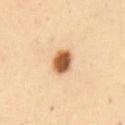The lesion was photographed on a routine skin check and not biopsied; there is no pathology result.
The lesion's longest dimension is about 2.5 mm.
On the abdomen.
This is a cross-polarized tile.
A female subject, about 50 years old.
A 15 mm close-up extracted from a 3D total-body photography capture.
An algorithmic analysis of the crop reported a lesion color around L≈50 a*≈19 b*≈35 in CIELAB, a lesion–skin lightness drop of about 18, and a normalized border contrast of about 12.5. The software also gave internal color variation of about 3.5 on a 0–10 scale.